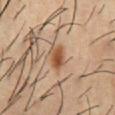Findings:
– diameter — ≈3.5 mm
– site — the front of the torso
– tile lighting — cross-polarized illumination
– TBP lesion metrics — a footprint of about 6 mm², an eccentricity of roughly 0.7, and a shape-asymmetry score of about 0.2 (0 = symmetric); a nevus-likeness score of about 100/100
– subject — male, in their mid-50s
– acquisition — total-body-photography crop, ~15 mm field of view